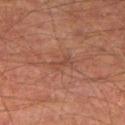Part of a total-body skin-imaging series; this lesion was reviewed on a skin check and was not flagged for biopsy.
The lesion is located on the right thigh.
A 15 mm close-up tile from a total-body photography series done for melanoma screening.
The recorded lesion diameter is about 2.5 mm.
The tile uses cross-polarized illumination.
The subject is a male aged approximately 65.